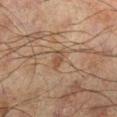follow-up: total-body-photography surveillance lesion; no biopsy | site: the left leg | image-analysis metrics: a lesion area of about 3 mm², an outline eccentricity of about 0.8 (0 = round, 1 = elongated), and a symmetry-axis asymmetry near 0.25; a color-variation rating of about 1/10 | illumination: cross-polarized | diameter: ~2.5 mm (longest diameter) | patient: male, roughly 60 years of age | image: ~15 mm crop, total-body skin-cancer survey.